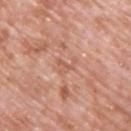{"biopsy_status": "not biopsied; imaged during a skin examination", "site": "upper back", "image": {"source": "total-body photography crop", "field_of_view_mm": 15}, "lesion_size": {"long_diameter_mm_approx": 2.5}, "patient": {"sex": "male", "age_approx": 70}, "lighting": "white-light", "automated_metrics": {"area_mm2_approx": 2.5, "border_irregularity_0_10": 7.5, "color_variation_0_10": 0.0}}